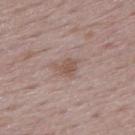Assessment:
This lesion was catalogued during total-body skin photography and was not selected for biopsy.
Background:
Located on the upper back. A roughly 15 mm field-of-view crop from a total-body skin photograph. The total-body-photography lesion software estimated a lesion area of about 4 mm², an eccentricity of roughly 0.7, and a shape-asymmetry score of about 0.45 (0 = symmetric). And it measured an average lesion color of about L≈53 a*≈17 b*≈24 (CIELAB), a lesion–skin lightness drop of about 8, and a normalized border contrast of about 6.5. And it measured a nevus-likeness score of about 15/100 and lesion-presence confidence of about 100/100. The tile uses white-light illumination. The recorded lesion diameter is about 3 mm. A female subject, roughly 50 years of age.